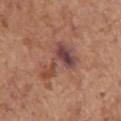The patient is a female in their mid-60s. The lesion is located on the left upper arm. A 15 mm crop from a total-body photograph taken for skin-cancer surveillance. Longest diameter approximately 5.5 mm. Captured under white-light illumination.